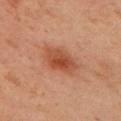biopsy_status: not biopsied; imaged during a skin examination
site: right upper arm
lesion_size:
  long_diameter_mm_approx: 4.0
automated_metrics:
  area_mm2_approx: 9.5
  eccentricity: 0.7
  shape_asymmetry: 0.15
  border_irregularity_0_10: 1.5
  color_variation_0_10: 4.5
  peripheral_color_asymmetry: 1.0
patient:
  sex: female
  age_approx: 40
image:
  source: total-body photography crop
  field_of_view_mm: 15